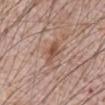This lesion was catalogued during total-body skin photography and was not selected for biopsy.
About 3 mm across.
The patient is a male roughly 70 years of age.
A 15 mm crop from a total-body photograph taken for skin-cancer surveillance.
The lesion is located on the abdomen.